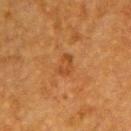Assessment: Captured during whole-body skin photography for melanoma surveillance; the lesion was not biopsied. Context: Cropped from a whole-body photographic skin survey; the tile spans about 15 mm. Approximately 2.5 mm at its widest. A female patient aged 53–57. Located on the upper back. Automated image analysis of the tile measured a mean CIELAB color near L≈39 a*≈23 b*≈36, a lesion–skin lightness drop of about 6, and a normalized lesion–skin contrast near 5.5. The software also gave a border-irregularity rating of about 3/10 and peripheral color asymmetry of about 0.5.